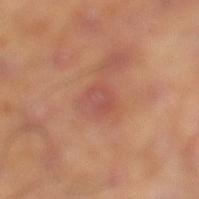<case>
<image>
  <source>total-body photography crop</source>
  <field_of_view_mm>15</field_of_view_mm>
</image>
<lighting>cross-polarized</lighting>
<automated_metrics>
  <color_variation_0_10>3.0</color_variation_0_10>
  <peripheral_color_asymmetry>1.0</peripheral_color_asymmetry>
  <nevus_likeness_0_100>0</nevus_likeness_0_100>
  <lesion_detection_confidence_0_100>100</lesion_detection_confidence_0_100>
</automated_metrics>
<site>left thigh</site>
<lesion_size>
  <long_diameter_mm_approx>2.5</long_diameter_mm_approx>
</lesion_size>
</case>